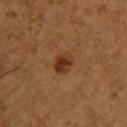image = ~15 mm tile from a whole-body skin photo | automated metrics = about 9 CIELAB-L* units darker than the surrounding skin and a normalized border contrast of about 9; a border-irregularity index near 1.5/10, a within-lesion color-variation index near 4.5/10, and peripheral color asymmetry of about 1.5 | size = ≈3 mm | subject = male, approximately 55 years of age | body site = the chest | lighting = cross-polarized.